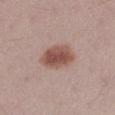Clinical impression: This lesion was catalogued during total-body skin photography and was not selected for biopsy. Background: Cropped from a whole-body photographic skin survey; the tile spans about 15 mm. The lesion's longest dimension is about 4.5 mm. The patient is a female aged 23–27. The lesion is on the left lower leg.